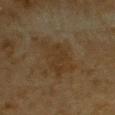Clinical impression: The lesion was photographed on a routine skin check and not biopsied; there is no pathology result. Acquisition and patient details: The patient is a male roughly 85 years of age or older. The lesion is located on the front of the torso. Cropped from a whole-body photographic skin survey; the tile spans about 15 mm. Automated tile analysis of the lesion measured a mean CIELAB color near L≈29 a*≈12 b*≈27, about 5 CIELAB-L* units darker than the surrounding skin, and a normalized border contrast of about 6. And it measured an automated nevus-likeness rating near 0 out of 100. About 5.5 mm across. The tile uses cross-polarized illumination.